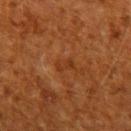notes: catalogued during a skin exam; not biopsied | image source: ~15 mm crop, total-body skin-cancer survey | body site: the right upper arm | lesion diameter: ≈2.5 mm | subject: male, in their 60s.